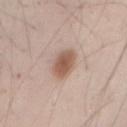Background: About 3.5 mm across. From the right forearm. A close-up tile cropped from a whole-body skin photograph, about 15 mm across. The total-body-photography lesion software estimated an average lesion color of about L≈56 a*≈19 b*≈28 (CIELAB), a lesion–skin lightness drop of about 13, and a lesion-to-skin contrast of about 8.5 (normalized; higher = more distinct). It also reported a color-variation rating of about 3/10. A male subject in their mid- to late 40s.